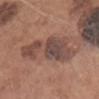Case summary:
- biopsy status — no biopsy performed (imaged during a skin exam)